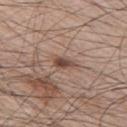Clinical impression:
The lesion was tiled from a total-body skin photograph and was not biopsied.
Acquisition and patient details:
Automated tile analysis of the lesion measured a lesion area of about 3.5 mm² and a shape eccentricity near 0.9. The software also gave a mean CIELAB color near L≈47 a*≈18 b*≈24. A lesion tile, about 15 mm wide, cut from a 3D total-body photograph. The tile uses white-light illumination. A male subject approximately 65 years of age. Approximately 3 mm at its widest. On the right upper arm.